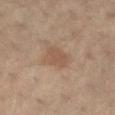follow-up = no biopsy performed (imaged during a skin exam)
tile lighting = cross-polarized illumination
diameter = ≈3 mm
imaging modality = 15 mm crop, total-body photography
subject = male, aged around 60
body site = the left thigh